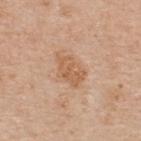Q: Was this lesion biopsied?
A: total-body-photography surveillance lesion; no biopsy
Q: Automated lesion metrics?
A: border irregularity of about 3 on a 0–10 scale and radial color variation of about 1
Q: What is the anatomic site?
A: the upper back
Q: What are the patient's age and sex?
A: male, approximately 65 years of age
Q: What is the lesion's diameter?
A: ~4 mm (longest diameter)
Q: What kind of image is this?
A: ~15 mm tile from a whole-body skin photo
Q: How was the tile lit?
A: white-light illumination